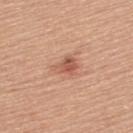The lesion was tiled from a total-body skin photograph and was not biopsied. Automated image analysis of the tile measured an area of roughly 5 mm², an eccentricity of roughly 0.65, and a symmetry-axis asymmetry near 0.45. And it measured an average lesion color of about L≈57 a*≈25 b*≈31 (CIELAB), about 11 CIELAB-L* units darker than the surrounding skin, and a normalized border contrast of about 7. A lesion tile, about 15 mm wide, cut from a 3D total-body photograph. Measured at roughly 3 mm in maximum diameter. Located on the upper back. A female subject about 55 years old. Captured under white-light illumination.